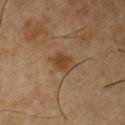Case summary:
* biopsy status: total-body-photography surveillance lesion; no biopsy
* image: total-body-photography crop, ~15 mm field of view
* subject: male, aged 53 to 57
* location: the chest
* lesion size: ~3.5 mm (longest diameter)
* illumination: cross-polarized